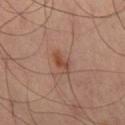– follow-up: no biopsy performed (imaged during a skin exam)
– anatomic site: the leg
– acquisition: ~15 mm tile from a whole-body skin photo